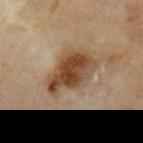Findings:
* biopsy status · no biopsy performed (imaged during a skin exam)
* acquisition · total-body-photography crop, ~15 mm field of view
* automated metrics · an area of roughly 18 mm² and two-axis asymmetry of about 0.25; a nevus-likeness score of about 100/100 and lesion-presence confidence of about 100/100
* location · the left upper arm
* patient · male, about 70 years old
* lesion diameter · about 6 mm